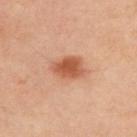No biopsy was performed on this lesion — it was imaged during a full skin examination and was not determined to be concerning. About 4 mm across. A male patient in their mid- to late 30s. Cropped from a whole-body photographic skin survey; the tile spans about 15 mm. Captured under cross-polarized illumination. An algorithmic analysis of the crop reported an average lesion color of about L≈54 a*≈26 b*≈34 (CIELAB), a lesion–skin lightness drop of about 12, and a normalized lesion–skin contrast near 8.5. The analysis additionally found a nevus-likeness score of about 95/100. Located on the upper back.